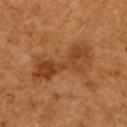notes: no biopsy performed (imaged during a skin exam)
anatomic site: the upper back
diameter: ~7.5 mm (longest diameter)
imaging modality: ~15 mm tile from a whole-body skin photo
automated lesion analysis: an average lesion color of about L≈44 a*≈25 b*≈39 (CIELAB), a lesion–skin lightness drop of about 9, and a normalized lesion–skin contrast near 7.5; a color-variation rating of about 5/10 and radial color variation of about 1.5; a nevus-likeness score of about 0/100 and a lesion-detection confidence of about 100/100
subject: female, aged 53–57